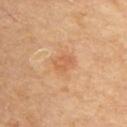Impression: No biopsy was performed on this lesion — it was imaged during a full skin examination and was not determined to be concerning. Background: A roughly 15 mm field-of-view crop from a total-body skin photograph. From the back. The patient is a male aged 83 or older.